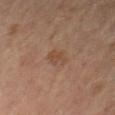No biopsy was performed on this lesion — it was imaged during a full skin examination and was not determined to be concerning.
Measured at roughly 3 mm in maximum diameter.
A close-up tile cropped from a whole-body skin photograph, about 15 mm across.
The patient is a female approximately 65 years of age.
Located on the left forearm.